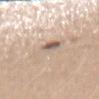Imaged during a routine full-body skin examination; the lesion was not biopsied and no histopathology is available. The subject is a female aged around 45. Located on the mid back. Cropped from a total-body skin-imaging series; the visible field is about 15 mm. Longest diameter approximately 6 mm.